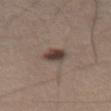Clinical summary: The recorded lesion diameter is about 3 mm. A 15 mm crop from a total-body photograph taken for skin-cancer surveillance. From the left thigh. The patient is a male aged around 55.